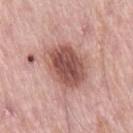The lesion was photographed on a routine skin check and not biopsied; there is no pathology result. Measured at roughly 5.5 mm in maximum diameter. The tile uses white-light illumination. A male subject approximately 55 years of age. The total-body-photography lesion software estimated a color-variation rating of about 5.5/10 and peripheral color asymmetry of about 1.5. And it measured lesion-presence confidence of about 100/100. On the right thigh. A close-up tile cropped from a whole-body skin photograph, about 15 mm across.